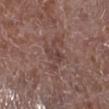| key | value |
|---|---|
| follow-up | no biopsy performed (imaged during a skin exam) |
| acquisition | 15 mm crop, total-body photography |
| illumination | white-light |
| patient | male, approximately 70 years of age |
| location | the right lower leg |